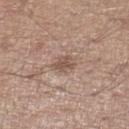The lesion was tiled from a total-body skin photograph and was not biopsied. On the leg. Captured under white-light illumination. A close-up tile cropped from a whole-body skin photograph, about 15 mm across. Measured at roughly 2.5 mm in maximum diameter. A male subject roughly 65 years of age.